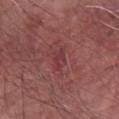Clinical impression:
The lesion was photographed on a routine skin check and not biopsied; there is no pathology result.
Clinical summary:
Imaged with white-light lighting. The lesion is located on the left forearm. A male patient aged around 60. Automated image analysis of the tile measured a footprint of about 3 mm², a shape eccentricity near 0.9, and a symmetry-axis asymmetry near 0.35. The software also gave an automated nevus-likeness rating near 0 out of 100. About 3 mm across. Cropped from a total-body skin-imaging series; the visible field is about 15 mm.